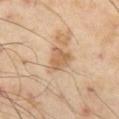Impression:
No biopsy was performed on this lesion — it was imaged during a full skin examination and was not determined to be concerning.
Context:
A male subject, aged approximately 55. A lesion tile, about 15 mm wide, cut from a 3D total-body photograph. Captured under cross-polarized illumination. The lesion is on the left thigh.